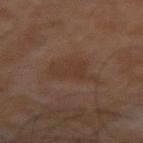Q: Is there a histopathology result?
A: no biopsy performed (imaged during a skin exam)
Q: How was the tile lit?
A: cross-polarized illumination
Q: How was this image acquired?
A: ~15 mm crop, total-body skin-cancer survey
Q: What is the anatomic site?
A: the abdomen
Q: Patient demographics?
A: male, aged approximately 60
Q: Lesion size?
A: ≈4.5 mm
Q: Automated lesion metrics?
A: a shape eccentricity near 0.75 and a shape-asymmetry score of about 0.4 (0 = symmetric)